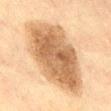Captured during whole-body skin photography for melanoma surveillance; the lesion was not biopsied.
The subject is a male aged 58 to 62.
A region of skin cropped from a whole-body photographic capture, roughly 15 mm wide.
Imaged with cross-polarized lighting.
Located on the front of the torso.